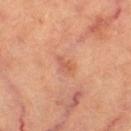Clinical impression:
The lesion was tiled from a total-body skin photograph and was not biopsied.
Clinical summary:
This image is a 15 mm lesion crop taken from a total-body photograph. Located on the leg. Measured at roughly 2.5 mm in maximum diameter. Automated tile analysis of the lesion measured a mean CIELAB color near L≈59 a*≈27 b*≈34. The analysis additionally found border irregularity of about 3 on a 0–10 scale, internal color variation of about 2.5 on a 0–10 scale, and radial color variation of about 1. A subject aged 58–62.